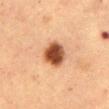{"biopsy_status": "not biopsied; imaged during a skin examination"}